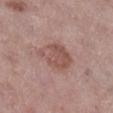<tbp_lesion>
<biopsy_status>not biopsied; imaged during a skin examination</biopsy_status>
<lesion_size>
  <long_diameter_mm_approx>4.5</long_diameter_mm_approx>
</lesion_size>
<automated_metrics>
  <area_mm2_approx>11.0</area_mm2_approx>
  <eccentricity>0.6</eccentricity>
  <shape_asymmetry>0.25</shape_asymmetry>
  <cielab_L>52</cielab_L>
  <cielab_a>21</cielab_a>
  <cielab_b>23</cielab_b>
  <vs_skin_darker_L>9.0</vs_skin_darker_L>
  <vs_skin_contrast_norm>6.5</vs_skin_contrast_norm>
  <border_irregularity_0_10>3.0</border_irregularity_0_10>
  <color_variation_0_10>3.5</color_variation_0_10>
  <peripheral_color_asymmetry>1.5</peripheral_color_asymmetry>
  <lesion_detection_confidence_0_100>100</lesion_detection_confidence_0_100>
</automated_metrics>
<image>
  <source>total-body photography crop</source>
  <field_of_view_mm>15</field_of_view_mm>
</image>
<patient>
  <sex>male</sex>
  <age_approx>70</age_approx>
</patient>
<lighting>white-light</lighting>
<site>right lower leg</site>
</tbp_lesion>